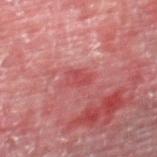notes: catalogued during a skin exam; not biopsied
image source: total-body-photography crop, ~15 mm field of view
anatomic site: the right thigh
subject: male, aged approximately 55